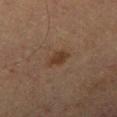The lesion was photographed on a routine skin check and not biopsied; there is no pathology result.
A region of skin cropped from a whole-body photographic capture, roughly 15 mm wide.
The lesion's longest dimension is about 2.5 mm.
Automated tile analysis of the lesion measured a classifier nevus-likeness of about 70/100 and a detector confidence of about 100 out of 100 that the crop contains a lesion.
A male subject, roughly 85 years of age or older.
Imaged with cross-polarized lighting.
The lesion is located on the leg.